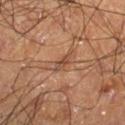Assessment: Part of a total-body skin-imaging series; this lesion was reviewed on a skin check and was not flagged for biopsy. Acquisition and patient details: The lesion is located on the right lower leg. This image is a 15 mm lesion crop taken from a total-body photograph. A male patient aged 58–62.